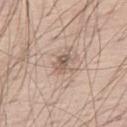Findings:
* workup — total-body-photography surveillance lesion; no biopsy
* patient — male, aged 58 to 62
* site — the right thigh
* lesion diameter — about 3 mm
* illumination — white-light
* acquisition — ~15 mm tile from a whole-body skin photo